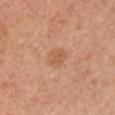Imaged during a routine full-body skin examination; the lesion was not biopsied and no histopathology is available.
The lesion's longest dimension is about 2.5 mm.
A male subject, aged 28–32.
The lesion-visualizer software estimated an area of roughly 5 mm², an eccentricity of roughly 0.5, and a shape-asymmetry score of about 0.2 (0 = symmetric). The analysis additionally found a classifier nevus-likeness of about 10/100 and lesion-presence confidence of about 100/100.
From the right upper arm.
A roughly 15 mm field-of-view crop from a total-body skin photograph.